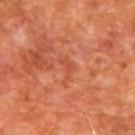workup: total-body-photography surveillance lesion; no biopsy
image: total-body-photography crop, ~15 mm field of view
diameter: about 3 mm
anatomic site: the upper back
image-analysis metrics: an average lesion color of about L≈49 a*≈32 b*≈37 (CIELAB) and about 6 CIELAB-L* units darker than the surrounding skin; a color-variation rating of about 0/10 and peripheral color asymmetry of about 0
patient: male, about 65 years old
tile lighting: cross-polarized illumination